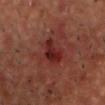Q: Was this lesion biopsied?
A: imaged on a skin check; not biopsied
Q: How was this image acquired?
A: ~15 mm crop, total-body skin-cancer survey
Q: What are the patient's age and sex?
A: male, approximately 55 years of age
Q: What is the anatomic site?
A: the head or neck
Q: What is the lesion's diameter?
A: ≈4 mm
Q: Automated lesion metrics?
A: an area of roughly 6.5 mm², an outline eccentricity of about 0.85 (0 = round, 1 = elongated), and a symmetry-axis asymmetry near 0.35; an average lesion color of about L≈26 a*≈28 b*≈24 (CIELAB) and roughly 9 lightness units darker than nearby skin; a border-irregularity index near 3.5/10, a color-variation rating of about 6.5/10, and peripheral color asymmetry of about 2.5